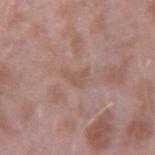biopsy_status: not biopsied; imaged during a skin examination
image:
  source: total-body photography crop
  field_of_view_mm: 15
automated_metrics:
  area_mm2_approx: 3.0
  eccentricity: 0.8
  shape_asymmetry: 0.55
  cielab_L: 54
  cielab_a: 18
  cielab_b: 25
  vs_skin_darker_L: 6.0
  border_irregularity_0_10: 5.5
  color_variation_0_10: 1.0
  peripheral_color_asymmetry: 0.5
site: right upper arm
lighting: white-light
patient:
  sex: male
  age_approx: 40
lesion_size:
  long_diameter_mm_approx: 2.5